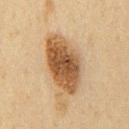Assessment:
No biopsy was performed on this lesion — it was imaged during a full skin examination and was not determined to be concerning.
Acquisition and patient details:
Cropped from a total-body skin-imaging series; the visible field is about 15 mm. A male subject, about 60 years old. The lesion is on the chest. The recorded lesion diameter is about 7.5 mm.